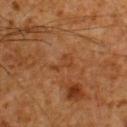• workup · no biopsy performed (imaged during a skin exam)
• lesion diameter · about 3 mm
• site · the upper back
• subject · male, in their 60s
• acquisition · 15 mm crop, total-body photography